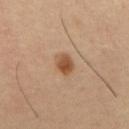Impression: Recorded during total-body skin imaging; not selected for excision or biopsy. Background: On the mid back. A 15 mm close-up tile from a total-body photography series done for melanoma screening. Imaged with cross-polarized lighting. The patient is a male aged approximately 60. The recorded lesion diameter is about 3 mm.